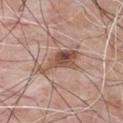<lesion>
  <image>
    <source>total-body photography crop</source>
    <field_of_view_mm>15</field_of_view_mm>
  </image>
  <site>chest</site>
  <lesion_size>
    <long_diameter_mm_approx>5.5</long_diameter_mm_approx>
  </lesion_size>
  <lighting>white-light</lighting>
  <automated_metrics>
    <cielab_L>52</cielab_L>
    <cielab_a>19</cielab_a>
    <cielab_b>27</cielab_b>
    <vs_skin_darker_L>12.0</vs_skin_darker_L>
    <vs_skin_contrast_norm>9.0</vs_skin_contrast_norm>
    <color_variation_0_10>7.0</color_variation_0_10>
    <peripheral_color_asymmetry>2.5</peripheral_color_asymmetry>
  </automated_metrics>
  <patient>
    <sex>male</sex>
    <age_approx>65</age_approx>
  </patient>
</lesion>